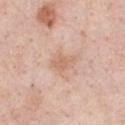{"biopsy_status": "not biopsied; imaged during a skin examination", "site": "chest", "lesion_size": {"long_diameter_mm_approx": 3.0}, "image": {"source": "total-body photography crop", "field_of_view_mm": 15}, "lighting": "white-light", "patient": {"sex": "male", "age_approx": 50}}